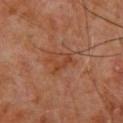{
  "biopsy_status": "not biopsied; imaged during a skin examination",
  "lesion_size": {
    "long_diameter_mm_approx": 3.5
  },
  "lighting": "cross-polarized",
  "site": "upper back",
  "image": {
    "source": "total-body photography crop",
    "field_of_view_mm": 15
  },
  "patient": {
    "sex": "male",
    "age_approx": 60
  },
  "automated_metrics": {
    "cielab_L": 38,
    "cielab_a": 23,
    "cielab_b": 30,
    "lesion_detection_confidence_0_100": 100
  }
}